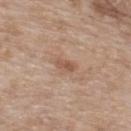biopsy_status: not biopsied; imaged during a skin examination
lesion_size:
  long_diameter_mm_approx: 2.5
image:
  source: total-body photography crop
  field_of_view_mm: 15
automated_metrics:
  cielab_L: 54
  cielab_a: 19
  cielab_b: 29
  vs_skin_darker_L: 9.0
  color_variation_0_10: 0.5
  peripheral_color_asymmetry: 0.0
  nevus_likeness_0_100: 0
  lesion_detection_confidence_0_100: 100
site: upper back
lighting: white-light
patient:
  sex: female
  age_approx: 75